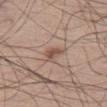Cropped from a total-body skin-imaging series; the visible field is about 15 mm.
The tile uses white-light illumination.
Located on the leg.
The patient is a male approximately 60 years of age.
An algorithmic analysis of the crop reported an average lesion color of about L≈52 a*≈18 b*≈26 (CIELAB), roughly 10 lightness units darker than nearby skin, and a lesion-to-skin contrast of about 7 (normalized; higher = more distinct). And it measured border irregularity of about 4 on a 0–10 scale, a color-variation rating of about 3.5/10, and radial color variation of about 1. The software also gave an automated nevus-likeness rating near 45 out of 100 and a lesion-detection confidence of about 100/100.
Approximately 2.5 mm at its widest.